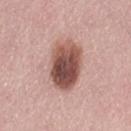Findings:
* follow-up · no biopsy performed (imaged during a skin exam)
* imaging modality · ~15 mm crop, total-body skin-cancer survey
* size · about 6 mm
* patient · female, aged approximately 30
* TBP lesion metrics · a border-irregularity index near 1.5/10, a within-lesion color-variation index near 8.5/10, and radial color variation of about 3
* anatomic site · the left thigh